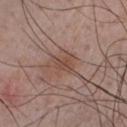{"patient": {"sex": "male", "age_approx": 55}, "site": "front of the torso", "automated_metrics": {"color_variation_0_10": 2.5, "peripheral_color_asymmetry": 1.0, "nevus_likeness_0_100": 10, "lesion_detection_confidence_0_100": 100}, "lighting": "white-light", "lesion_size": {"long_diameter_mm_approx": 3.0}, "image": {"source": "total-body photography crop", "field_of_view_mm": 15}}